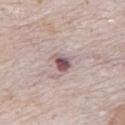Assessment:
Recorded during total-body skin imaging; not selected for excision or biopsy.
Acquisition and patient details:
Automated image analysis of the tile measured a lesion area of about 4 mm² and a shape eccentricity near 0.75. The software also gave a within-lesion color-variation index near 5/10 and peripheral color asymmetry of about 1.5. A 15 mm close-up extracted from a 3D total-body photography capture. The subject is a male about 80 years old. The tile uses white-light illumination. The lesion is located on the back. About 2.5 mm across.